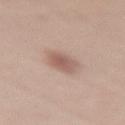No biopsy was performed on this lesion — it was imaged during a full skin examination and was not determined to be concerning.
Automated tile analysis of the lesion measured a lesion area of about 6.5 mm², a shape eccentricity near 0.8, and two-axis asymmetry of about 0.15. It also reported a lesion color around L≈58 a*≈18 b*≈25 in CIELAB, roughly 11 lightness units darker than nearby skin, and a lesion-to-skin contrast of about 7 (normalized; higher = more distinct). It also reported internal color variation of about 3 on a 0–10 scale. The analysis additionally found an automated nevus-likeness rating near 85 out of 100 and a detector confidence of about 100 out of 100 that the crop contains a lesion.
A 15 mm crop from a total-body photograph taken for skin-cancer surveillance.
The subject is a female aged 38 to 42.
Approximately 4 mm at its widest.
The tile uses white-light illumination.
Located on the right thigh.